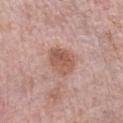A 15 mm close-up tile from a total-body photography series done for melanoma screening. The tile uses white-light illumination. The lesion is on the left forearm. A female patient, in their mid- to late 60s.